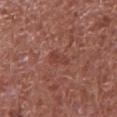Part of a total-body skin-imaging series; this lesion was reviewed on a skin check and was not flagged for biopsy. The lesion-visualizer software estimated a lesion color around L≈42 a*≈26 b*≈26 in CIELAB, about 6 CIELAB-L* units darker than the surrounding skin, and a normalized lesion–skin contrast near 5. It also reported a nevus-likeness score of about 0/100 and a lesion-detection confidence of about 100/100. This image is a 15 mm lesion crop taken from a total-body photograph. On the abdomen. A male patient in their mid- to late 60s. The tile uses white-light illumination. The recorded lesion diameter is about 3 mm.